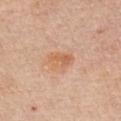Part of a total-body skin-imaging series; this lesion was reviewed on a skin check and was not flagged for biopsy.
A close-up tile cropped from a whole-body skin photograph, about 15 mm across.
The total-body-photography lesion software estimated a lesion area of about 5.5 mm², an eccentricity of roughly 0.8, and two-axis asymmetry of about 0.2. The analysis additionally found a border-irregularity rating of about 2/10, internal color variation of about 4 on a 0–10 scale, and peripheral color asymmetry of about 1.5.
A male patient, roughly 65 years of age.
The lesion is on the chest.
Imaged with white-light lighting.
Measured at roughly 3 mm in maximum diameter.